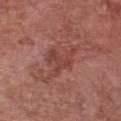This lesion was catalogued during total-body skin photography and was not selected for biopsy. Longest diameter approximately 5 mm. This is a white-light tile. A roughly 15 mm field-of-view crop from a total-body skin photograph. An algorithmic analysis of the crop reported a footprint of about 8.5 mm², an eccentricity of roughly 0.7, and two-axis asymmetry of about 0.6. The software also gave a lesion–skin lightness drop of about 7 and a lesion-to-skin contrast of about 5.5 (normalized; higher = more distinct). The software also gave an automated nevus-likeness rating near 0 out of 100. The lesion is on the front of the torso. The subject is a male aged 78 to 82.